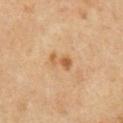Part of a total-body skin-imaging series; this lesion was reviewed on a skin check and was not flagged for biopsy.
A 15 mm close-up extracted from a 3D total-body photography capture.
From the right upper arm.
The subject is a male approximately 55 years of age.
Longest diameter approximately 3 mm.